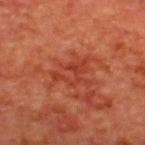Clinical impression:
Recorded during total-body skin imaging; not selected for excision or biopsy.
Background:
Captured under cross-polarized illumination. The lesion is located on the back. A 15 mm crop from a total-body photograph taken for skin-cancer surveillance. The lesion-visualizer software estimated a shape eccentricity near 0.8 and a symmetry-axis asymmetry near 0.6. It also reported internal color variation of about 0.5 on a 0–10 scale and peripheral color asymmetry of about 0. The subject is a male in their mid- to late 60s. Approximately 4 mm at its widest.